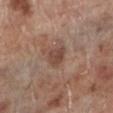Q: Was this lesion biopsied?
A: imaged on a skin check; not biopsied
Q: What is the lesion's diameter?
A: ≈2.5 mm
Q: What is the imaging modality?
A: total-body-photography crop, ~15 mm field of view
Q: What did automated image analysis measure?
A: a lesion color around L≈45 a*≈19 b*≈25 in CIELAB and a normalized lesion–skin contrast near 7
Q: Patient demographics?
A: male, aged approximately 70
Q: How was the tile lit?
A: white-light illumination
Q: What is the anatomic site?
A: the left lower leg